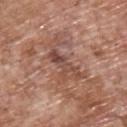Clinical impression: The lesion was photographed on a routine skin check and not biopsied; there is no pathology result. Clinical summary: A 15 mm crop from a total-body photograph taken for skin-cancer surveillance. A male patient, roughly 70 years of age. Automated image analysis of the tile measured a color-variation rating of about 8/10 and a peripheral color-asymmetry measure near 2.5. It also reported a lesion-detection confidence of about 80/100. This is a white-light tile. From the upper back.